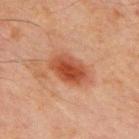follow-up: catalogued during a skin exam; not biopsied
patient: male, approximately 70 years of age
site: the front of the torso
acquisition: ~15 mm tile from a whole-body skin photo
lesion diameter: about 4.5 mm
lighting: cross-polarized illumination